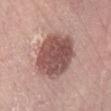Captured during whole-body skin photography for melanoma surveillance; the lesion was not biopsied.
This image is a 15 mm lesion crop taken from a total-body photograph.
On the right lower leg.
Imaged with white-light lighting.
An algorithmic analysis of the crop reported a mean CIELAB color near L≈51 a*≈22 b*≈22 and a lesion–skin lightness drop of about 15. The software also gave an automated nevus-likeness rating near 25 out of 100.
A female patient aged approximately 55.